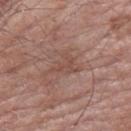Assessment:
Part of a total-body skin-imaging series; this lesion was reviewed on a skin check and was not flagged for biopsy.
Clinical summary:
Automated image analysis of the tile measured a border-irregularity rating of about 7.5/10, a color-variation rating of about 0.5/10, and a peripheral color-asymmetry measure near 0. The analysis additionally found a classifier nevus-likeness of about 0/100 and a detector confidence of about 95 out of 100 that the crop contains a lesion. The recorded lesion diameter is about 3.5 mm. The lesion is located on the left forearm. A male subject, aged 58–62. A 15 mm close-up tile from a total-body photography series done for melanoma screening.